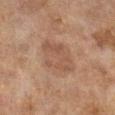| feature | finding |
|---|---|
| follow-up | total-body-photography surveillance lesion; no biopsy |
| automated metrics | an area of roughly 16 mm², an eccentricity of roughly 0.65, and two-axis asymmetry of about 0.2; a border-irregularity index near 2/10, a color-variation rating of about 2.5/10, and peripheral color asymmetry of about 1 |
| subject | female, aged 58–62 |
| tile lighting | cross-polarized illumination |
| acquisition | ~15 mm crop, total-body skin-cancer survey |
| location | the right lower leg |